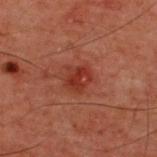Findings:
– illumination · cross-polarized illumination
– subject · male, aged approximately 60
– lesion diameter · ~3 mm (longest diameter)
– site · the upper back
– image source · ~15 mm tile from a whole-body skin photo